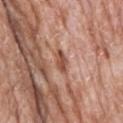{
  "biopsy_status": "not biopsied; imaged during a skin examination",
  "image": {
    "source": "total-body photography crop",
    "field_of_view_mm": 15
  },
  "site": "head or neck",
  "patient": {
    "sex": "male",
    "age_approx": 75
  }
}